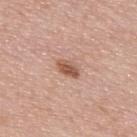A close-up tile cropped from a whole-body skin photograph, about 15 mm across. Approximately 3 mm at its widest. The lesion is located on the upper back. This is a white-light tile. A male subject aged approximately 55.